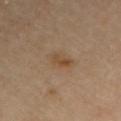The lesion was photographed on a routine skin check and not biopsied; there is no pathology result. A 15 mm close-up tile from a total-body photography series done for melanoma screening. The lesion is located on the right upper arm. Imaged with cross-polarized lighting.